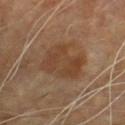notes = catalogued during a skin exam; not biopsied
size = ~5 mm (longest diameter)
image source = ~15 mm tile from a whole-body skin photo
location = the chest
subject = male, about 60 years old
lighting = cross-polarized illumination